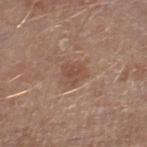Impression: No biopsy was performed on this lesion — it was imaged during a full skin examination and was not determined to be concerning. Clinical summary: This is a white-light tile. On the right thigh. A male patient in their mid- to late 60s. A roughly 15 mm field-of-view crop from a total-body skin photograph.